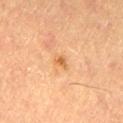– follow-up · total-body-photography surveillance lesion; no biopsy
– imaging modality · 15 mm crop, total-body photography
– lesion size · about 2 mm
– tile lighting · cross-polarized
– patient · male, in their mid-60s
– site · the right thigh